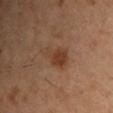Q: Was this lesion biopsied?
A: catalogued during a skin exam; not biopsied
Q: What are the patient's age and sex?
A: male, aged around 55
Q: What kind of image is this?
A: 15 mm crop, total-body photography
Q: Where on the body is the lesion?
A: the chest